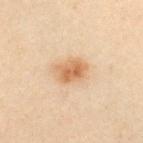– follow-up: total-body-photography surveillance lesion; no biopsy
– site: the chest
– image source: total-body-photography crop, ~15 mm field of view
– patient: female, aged 28–32
– illumination: cross-polarized